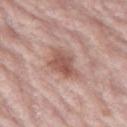workup — imaged on a skin check; not biopsied | subject — female, aged approximately 70 | lighting — white-light illumination | TBP lesion metrics — a mean CIELAB color near L≈54 a*≈22 b*≈25, roughly 11 lightness units darker than nearby skin, and a lesion-to-skin contrast of about 8 (normalized; higher = more distinct) | body site — the left thigh | image source — ~15 mm tile from a whole-body skin photo.